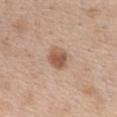Image and clinical context:
The tile uses white-light illumination. Automated image analysis of the tile measured a mean CIELAB color near L≈56 a*≈19 b*≈31 and a normalized border contrast of about 8.5. It also reported a color-variation rating of about 5/10. And it measured a lesion-detection confidence of about 100/100. Located on the chest. This image is a 15 mm lesion crop taken from a total-body photograph. The patient is a female aged around 40.